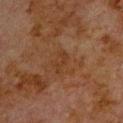anatomic site — the upper back; lighting — cross-polarized illumination; acquisition — ~15 mm tile from a whole-body skin photo; lesion size — about 3 mm; patient — male, in their 80s.